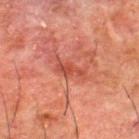Part of a total-body skin-imaging series; this lesion was reviewed on a skin check and was not flagged for biopsy.
Longest diameter approximately 3 mm.
A 15 mm close-up tile from a total-body photography series done for melanoma screening.
The lesion is on the upper back.
The patient is a male aged 48–52.
Imaged with cross-polarized lighting.